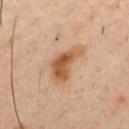Captured during whole-body skin photography for melanoma surveillance; the lesion was not biopsied.
Imaged with cross-polarized lighting.
Located on the upper back.
About 5.5 mm across.
Automated image analysis of the tile measured a lesion area of about 11 mm², a shape eccentricity near 0.85, and two-axis asymmetry of about 0.45. It also reported a lesion color around L≈55 a*≈21 b*≈36 in CIELAB, roughly 11 lightness units darker than nearby skin, and a normalized lesion–skin contrast near 9. The software also gave a classifier nevus-likeness of about 95/100 and lesion-presence confidence of about 100/100.
A male patient, approximately 40 years of age.
This image is a 15 mm lesion crop taken from a total-body photograph.